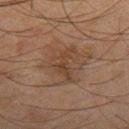| key | value |
|---|---|
| workup | total-body-photography surveillance lesion; no biopsy |
| patient | male, aged around 65 |
| anatomic site | the right thigh |
| illumination | cross-polarized illumination |
| image-analysis metrics | an outline eccentricity of about 0.9 (0 = round, 1 = elongated) and two-axis asymmetry of about 0.65 |
| size | about 4 mm |
| acquisition | ~15 mm crop, total-body skin-cancer survey |